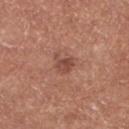Assessment: Imaged during a routine full-body skin examination; the lesion was not biopsied and no histopathology is available. Image and clinical context: A male patient aged 68–72. The lesion is on the left lower leg. The tile uses white-light illumination. A 15 mm close-up extracted from a 3D total-body photography capture.